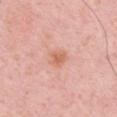Q: Is there a histopathology result?
A: no biopsy performed (imaged during a skin exam)
Q: What lighting was used for the tile?
A: white-light illumination
Q: What is the imaging modality?
A: 15 mm crop, total-body photography
Q: How large is the lesion?
A: about 2.5 mm
Q: Patient demographics?
A: male, approximately 50 years of age
Q: Lesion location?
A: the left upper arm